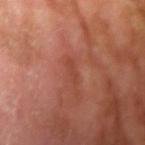Recorded during total-body skin imaging; not selected for excision or biopsy. Measured at roughly 3 mm in maximum diameter. A male subject, in their mid-50s. The lesion is on the right upper arm. Captured under cross-polarized illumination. A 15 mm close-up extracted from a 3D total-body photography capture. The total-body-photography lesion software estimated border irregularity of about 4 on a 0–10 scale, internal color variation of about 0 on a 0–10 scale, and radial color variation of about 0. It also reported a nevus-likeness score of about 0/100 and a lesion-detection confidence of about 95/100.